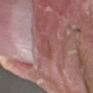Clinical impression:
Captured during whole-body skin photography for melanoma surveillance; the lesion was not biopsied.
Context:
A male subject, aged 38 to 42. From the right forearm. The recorded lesion diameter is about 3 mm. Imaged with white-light lighting. A 15 mm close-up extracted from a 3D total-body photography capture.